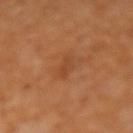Recorded during total-body skin imaging; not selected for excision or biopsy.